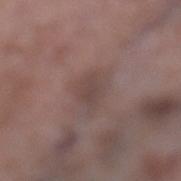Q: Was a biopsy performed?
A: catalogued during a skin exam; not biopsied
Q: What is the imaging modality?
A: total-body-photography crop, ~15 mm field of view
Q: Who is the patient?
A: female, about 70 years old
Q: Where on the body is the lesion?
A: the left lower leg